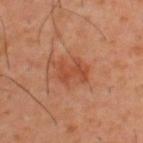The lesion was photographed on a routine skin check and not biopsied; there is no pathology result. From the upper back. Imaged with cross-polarized lighting. A 15 mm crop from a total-body photograph taken for skin-cancer surveillance. A male patient, aged around 40. About 3.5 mm across.